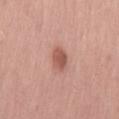Part of a total-body skin-imaging series; this lesion was reviewed on a skin check and was not flagged for biopsy. The total-body-photography lesion software estimated a lesion area of about 5 mm² and a symmetry-axis asymmetry near 0.2. It also reported border irregularity of about 2 on a 0–10 scale, internal color variation of about 2.5 on a 0–10 scale, and radial color variation of about 1. Approximately 3 mm at its widest. A male subject, about 55 years old. Cropped from a whole-body photographic skin survey; the tile spans about 15 mm. The lesion is on the lower back.